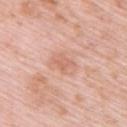Q: Was a biopsy performed?
A: imaged on a skin check; not biopsied
Q: What kind of image is this?
A: 15 mm crop, total-body photography
Q: Lesion location?
A: the upper back
Q: What are the patient's age and sex?
A: female, in their mid- to late 60s